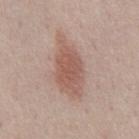workup: imaged on a skin check; not biopsied | anatomic site: the abdomen | imaging modality: ~15 mm crop, total-body skin-cancer survey | patient: male, aged 58 to 62.